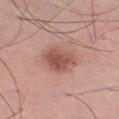workup: total-body-photography surveillance lesion; no biopsy | size: ~4 mm (longest diameter) | subject: male, aged approximately 50 | image: total-body-photography crop, ~15 mm field of view | site: the right lower leg | image-analysis metrics: a lesion color around L≈53 a*≈24 b*≈25 in CIELAB, roughly 12 lightness units darker than nearby skin, and a lesion-to-skin contrast of about 8 (normalized; higher = more distinct); a border-irregularity index near 2/10 and a within-lesion color-variation index near 4/10; a classifier nevus-likeness of about 85/100 and a lesion-detection confidence of about 100/100.